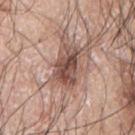Notes:
– biopsy status — catalogued during a skin exam; not biopsied
– location — the left arm
– patient — male, approximately 70 years of age
– image source — total-body-photography crop, ~15 mm field of view
– tile lighting — white-light
– diameter — ≈5 mm